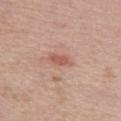Located on the chest. A roughly 15 mm field-of-view crop from a total-body skin photograph. A male subject, approximately 60 years of age.